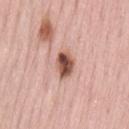Image and clinical context: A female patient, aged around 70. A lesion tile, about 15 mm wide, cut from a 3D total-body photograph. The lesion is located on the lower back.